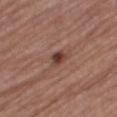| feature | finding |
|---|---|
| follow-up | imaged on a skin check; not biopsied |
| subject | female, aged 73–77 |
| body site | the right thigh |
| image | ~15 mm crop, total-body skin-cancer survey |